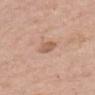Findings:
- follow-up — no biopsy performed (imaged during a skin exam)
- acquisition — total-body-photography crop, ~15 mm field of view
- site — the upper back
- subject — female, in their mid-60s
- TBP lesion metrics — an area of roughly 4 mm², an outline eccentricity of about 0.55 (0 = round, 1 = elongated), and a shape-asymmetry score of about 0.25 (0 = symmetric); a lesion–skin lightness drop of about 9 and a normalized border contrast of about 6.5; an automated nevus-likeness rating near 15 out of 100 and lesion-presence confidence of about 100/100